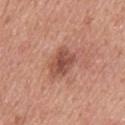No biopsy was performed on this lesion — it was imaged during a full skin examination and was not determined to be concerning.
Located on the back.
A male subject aged 58 to 62.
Cropped from a total-body skin-imaging series; the visible field is about 15 mm.